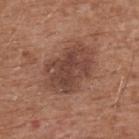Background: Located on the back. The recorded lesion diameter is about 6.5 mm. The tile uses white-light illumination. Cropped from a whole-body photographic skin survey; the tile spans about 15 mm. A male patient roughly 75 years of age.